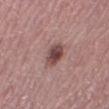Impression:
The lesion was tiled from a total-body skin photograph and was not biopsied.
Context:
Imaged with white-light lighting. The lesion-visualizer software estimated a lesion color around L≈46 a*≈21 b*≈20 in CIELAB, a lesion–skin lightness drop of about 13, and a normalized border contrast of about 10. And it measured a border-irregularity index near 1.5/10, internal color variation of about 4 on a 0–10 scale, and a peripheral color-asymmetry measure near 1.5. A female subject aged approximately 50. Longest diameter approximately 3 mm. Located on the right thigh. A close-up tile cropped from a whole-body skin photograph, about 15 mm across.